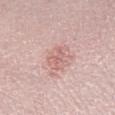biopsy status = no biopsy performed (imaged during a skin exam)
image = 15 mm crop, total-body photography
lighting = white-light illumination
subject = male, aged 48 to 52
size = about 3 mm
body site = the right lower leg